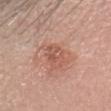This lesion was catalogued during total-body skin photography and was not selected for biopsy. The lesion-visualizer software estimated a mean CIELAB color near L≈55 a*≈24 b*≈29, roughly 8 lightness units darker than nearby skin, and a normalized lesion–skin contrast near 5.5. The analysis additionally found a border-irregularity index near 4.5/10, internal color variation of about 4.5 on a 0–10 scale, and peripheral color asymmetry of about 1.5. On the head or neck. Longest diameter approximately 3.5 mm. A female patient, about 30 years old. A lesion tile, about 15 mm wide, cut from a 3D total-body photograph. The tile uses white-light illumination.